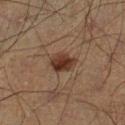A male patient, aged 48–52.
Approximately 3 mm at its widest.
From the left lower leg.
The total-body-photography lesion software estimated an eccentricity of roughly 0.6 and a symmetry-axis asymmetry near 0.2. The software also gave a classifier nevus-likeness of about 95/100.
This image is a 15 mm lesion crop taken from a total-body photograph.